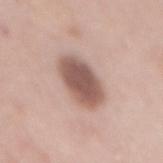Imaged during a routine full-body skin examination; the lesion was not biopsied and no histopathology is available.
The patient is a female about 50 years old.
The lesion is on the mid back.
The recorded lesion diameter is about 6 mm.
A 15 mm close-up extracted from a 3D total-body photography capture.
Imaged with white-light lighting.